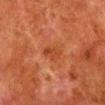biopsy_status: not biopsied; imaged during a skin examination
patient:
  sex: male
  age_approx: 80
lighting: cross-polarized
lesion_size:
  long_diameter_mm_approx: 2.5
site: left lower leg
image:
  source: total-body photography crop
  field_of_view_mm: 15